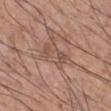| key | value |
|---|---|
| notes | imaged on a skin check; not biopsied |
| acquisition | 15 mm crop, total-body photography |
| subject | male, in their mid-50s |
| body site | the right upper arm |